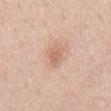notes — catalogued during a skin exam; not biopsied | imaging modality — total-body-photography crop, ~15 mm field of view | body site — the mid back | illumination — white-light | patient — female, about 40 years old | diameter — ≈3.5 mm.